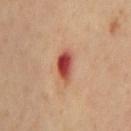| field | value |
|---|---|
| subject | male, aged around 60 |
| diameter | ≈3.5 mm |
| anatomic site | the mid back |
| automated lesion analysis | a lesion color around L≈48 a*≈31 b*≈30 in CIELAB, roughly 15 lightness units darker than nearby skin, and a normalized border contrast of about 11; a classifier nevus-likeness of about 0/100 and lesion-presence confidence of about 100/100 |
| lighting | cross-polarized |
| acquisition | ~15 mm tile from a whole-body skin photo |